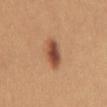Clinical impression: No biopsy was performed on this lesion — it was imaged during a full skin examination and was not determined to be concerning. Acquisition and patient details: From the abdomen. A 15 mm close-up extracted from a 3D total-body photography capture. The tile uses white-light illumination. Approximately 4 mm at its widest. The subject is a female in their mid- to late 20s. An algorithmic analysis of the crop reported a lesion area of about 7 mm² and two-axis asymmetry of about 0.2. The analysis additionally found a classifier nevus-likeness of about 100/100 and a lesion-detection confidence of about 100/100.